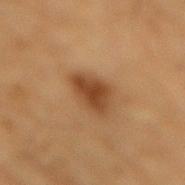Recorded during total-body skin imaging; not selected for excision or biopsy.